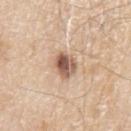Notes:
– biopsy status — no biopsy performed (imaged during a skin exam)
– tile lighting — white-light
– image source — ~15 mm tile from a whole-body skin photo
– site — the left upper arm
– subject — male, approximately 80 years of age
– diameter — ~3 mm (longest diameter)
– automated metrics — a lesion area of about 6 mm² and a shape eccentricity near 0.35; a mean CIELAB color near L≈56 a*≈19 b*≈28, roughly 17 lightness units darker than nearby skin, and a lesion-to-skin contrast of about 10.5 (normalized; higher = more distinct); a nevus-likeness score of about 90/100 and a detector confidence of about 100 out of 100 that the crop contains a lesion